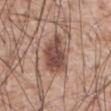Assessment: Imaged during a routine full-body skin examination; the lesion was not biopsied and no histopathology is available. Acquisition and patient details: A male subject roughly 60 years of age. An algorithmic analysis of the crop reported a lesion area of about 12 mm² and a shape-asymmetry score of about 0.25 (0 = symmetric). The software also gave an average lesion color of about L≈47 a*≈20 b*≈24 (CIELAB), about 14 CIELAB-L* units darker than the surrounding skin, and a lesion-to-skin contrast of about 10 (normalized; higher = more distinct). The software also gave a nevus-likeness score of about 90/100 and a lesion-detection confidence of about 100/100. This is a white-light tile. Longest diameter approximately 5 mm. A 15 mm crop from a total-body photograph taken for skin-cancer surveillance. Located on the abdomen.